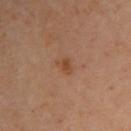Impression: Recorded during total-body skin imaging; not selected for excision or biopsy. Background: Imaged with cross-polarized lighting. A region of skin cropped from a whole-body photographic capture, roughly 15 mm wide. A female subject aged approximately 60. Measured at roughly 2.5 mm in maximum diameter. The lesion is on the right upper arm.